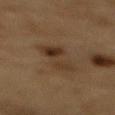  biopsy_status: not biopsied; imaged during a skin examination
  patient:
    sex: male
    age_approx: 85+
  site: mid back
  lesion_size:
    long_diameter_mm_approx: 5.5
  lighting: cross-polarized
  automated_metrics:
    vs_skin_darker_L: 7.0
    vs_skin_contrast_norm: 7.0
    border_irregularity_0_10: 3.0
    peripheral_color_asymmetry: 2.5
    nevus_likeness_0_100: 100
    lesion_detection_confidence_0_100: 100
  image:
    source: total-body photography crop
    field_of_view_mm: 15